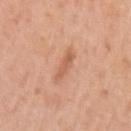Assessment:
This lesion was catalogued during total-body skin photography and was not selected for biopsy.
Context:
Located on the left upper arm. A 15 mm close-up tile from a total-body photography series done for melanoma screening. The patient is a female approximately 45 years of age. Imaged with white-light lighting.